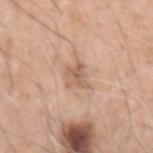Impression: Imaged during a routine full-body skin examination; the lesion was not biopsied and no histopathology is available. Background: The patient is a male aged 58 to 62. On the left upper arm. Imaged with white-light lighting. A 15 mm close-up tile from a total-body photography series done for melanoma screening.